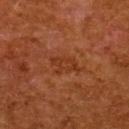Imaged during a routine full-body skin examination; the lesion was not biopsied and no histopathology is available.
A female patient, roughly 50 years of age.
An algorithmic analysis of the crop reported a mean CIELAB color near L≈29 a*≈23 b*≈30 and a normalized border contrast of about 5. It also reported a classifier nevus-likeness of about 0/100 and lesion-presence confidence of about 100/100.
The lesion is located on the back.
Captured under cross-polarized illumination.
This image is a 15 mm lesion crop taken from a total-body photograph.